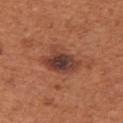Imaged during a routine full-body skin examination; the lesion was not biopsied and no histopathology is available.
From the right upper arm.
Measured at roughly 4.5 mm in maximum diameter.
A roughly 15 mm field-of-view crop from a total-body skin photograph.
A female patient, in their mid-60s.
This is a white-light tile.